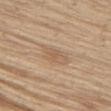Clinical impression: Captured during whole-body skin photography for melanoma surveillance; the lesion was not biopsied. Acquisition and patient details: On the front of the torso. Approximately 3.5 mm at its widest. Automated image analysis of the tile measured a border-irregularity index near 3/10, a within-lesion color-variation index near 2/10, and peripheral color asymmetry of about 0.5. And it measured an automated nevus-likeness rating near 0 out of 100 and a detector confidence of about 100 out of 100 that the crop contains a lesion. A male patient approximately 70 years of age. A 15 mm crop from a total-body photograph taken for skin-cancer surveillance.